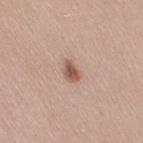biopsy status — imaged on a skin check; not biopsied | body site — the right thigh | size — ≈2.5 mm | automated lesion analysis — a mean CIELAB color near L≈56 a*≈20 b*≈26, roughly 12 lightness units darker than nearby skin, and a normalized border contrast of about 8; a classifier nevus-likeness of about 95/100 | patient — female, aged around 25 | image — total-body-photography crop, ~15 mm field of view | illumination — white-light.